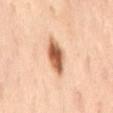Imaged during a routine full-body skin examination; the lesion was not biopsied and no histopathology is available.